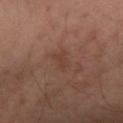biopsy status = total-body-photography surveillance lesion; no biopsy | patient = male, about 40 years old | image = ~15 mm crop, total-body skin-cancer survey | anatomic site = the arm.